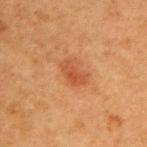The lesion was tiled from a total-body skin photograph and was not biopsied. The lesion-visualizer software estimated an average lesion color of about L≈45 a*≈26 b*≈35 (CIELAB) and a normalized border contrast of about 6. And it measured a within-lesion color-variation index near 3/10 and a peripheral color-asymmetry measure near 1. The software also gave a classifier nevus-likeness of about 40/100. The lesion is located on the right upper arm. A region of skin cropped from a whole-body photographic capture, roughly 15 mm wide. Measured at roughly 3 mm in maximum diameter. The subject is a female in their 40s. Captured under cross-polarized illumination.